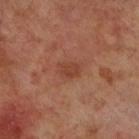imaging modality: total-body-photography crop, ~15 mm field of view | diameter: ~3 mm (longest diameter) | image-analysis metrics: a lesion area of about 5 mm² and a shape-asymmetry score of about 0.3 (0 = symmetric); a normalized lesion–skin contrast near 5.5; a within-lesion color-variation index near 2.5/10; an automated nevus-likeness rating near 0 out of 100 and a detector confidence of about 100 out of 100 that the crop contains a lesion | site: the leg | lighting: cross-polarized | patient: male, aged 68–72.